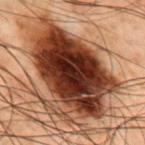  site: chest
  automated_metrics:
    border_irregularity_0_10: 2.5
    color_variation_0_10: 8.0
    peripheral_color_asymmetry: 2.5
    nevus_likeness_0_100: 45
    lesion_detection_confidence_0_100: 100
  lesion_size:
    long_diameter_mm_approx: 10.5
  lighting: cross-polarized
  image:
    source: total-body photography crop
    field_of_view_mm: 15
  patient:
    sex: male
    age_approx: 70
  diagnosis:
    histopathology: dysplastic (Clark) nevus
    malignancy: benign
    taxonomic_path:
      - Benign
      - Benign melanocytic proliferations
      - Nevus
      - Nevus, Atypical, Dysplastic, or Clark